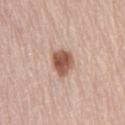Recorded during total-body skin imaging; not selected for excision or biopsy. Imaged with white-light lighting. The recorded lesion diameter is about 3.5 mm. A 15 mm close-up tile from a total-body photography series done for melanoma screening. A male subject aged 63–67. On the back.